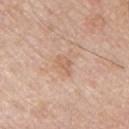| key | value |
|---|---|
| workup | catalogued during a skin exam; not biopsied |